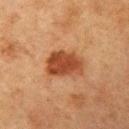| field | value |
|---|---|
| follow-up | total-body-photography surveillance lesion; no biopsy |
| illumination | cross-polarized |
| location | the left upper arm |
| TBP lesion metrics | border irregularity of about 2.5 on a 0–10 scale, internal color variation of about 3 on a 0–10 scale, and radial color variation of about 1 |
| size | ≈4.5 mm |
| image | ~15 mm crop, total-body skin-cancer survey |
| subject | female, aged around 55 |